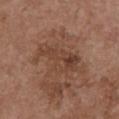Impression:
This lesion was catalogued during total-body skin photography and was not selected for biopsy.
Background:
A 15 mm close-up extracted from a 3D total-body photography capture. The tile uses white-light illumination. Approximately 6.5 mm at its widest. A female patient aged around 65. An algorithmic analysis of the crop reported a lesion area of about 15 mm² and a shape-asymmetry score of about 0.45 (0 = symmetric). The analysis additionally found a mean CIELAB color near L≈42 a*≈20 b*≈28 and a lesion-to-skin contrast of about 6.5 (normalized; higher = more distinct). The analysis additionally found radial color variation of about 2. On the chest.